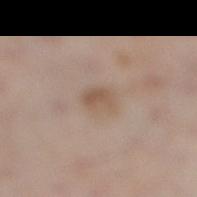Clinical impression: The lesion was photographed on a routine skin check and not biopsied; there is no pathology result. Clinical summary: Measured at roughly 2.5 mm in maximum diameter. The subject is a male aged 58 to 62. A 15 mm close-up tile from a total-body photography series done for melanoma screening. Captured under white-light illumination. Automated tile analysis of the lesion measured an average lesion color of about L≈54 a*≈16 b*≈27 (CIELAB), roughly 8 lightness units darker than nearby skin, and a normalized border contrast of about 6.5. The analysis additionally found a within-lesion color-variation index near 2.5/10 and radial color variation of about 1. Located on the right lower leg.